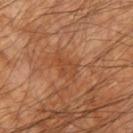Findings:
* follow-up · imaged on a skin check; not biopsied
* image source · ~15 mm crop, total-body skin-cancer survey
* automated metrics · a lesion area of about 5.5 mm², a shape eccentricity near 0.85, and a symmetry-axis asymmetry near 0.4; a lesion-detection confidence of about 100/100
* diameter · ~3.5 mm (longest diameter)
* site · the arm
* subject · male, aged around 60
* lighting · cross-polarized illumination